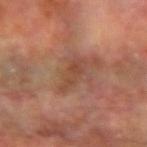The lesion was tiled from a total-body skin photograph and was not biopsied.
This is a cross-polarized tile.
A 15 mm crop from a total-body photograph taken for skin-cancer surveillance.
The lesion-visualizer software estimated a lesion color around L≈44 a*≈22 b*≈30 in CIELAB and about 7 CIELAB-L* units darker than the surrounding skin.
From the left forearm.
A male patient aged 68–72.